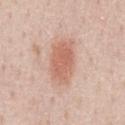Q: Was a biopsy performed?
A: catalogued during a skin exam; not biopsied
Q: Illumination type?
A: white-light
Q: How was this image acquired?
A: 15 mm crop, total-body photography
Q: Lesion location?
A: the abdomen
Q: Patient demographics?
A: male, aged 58 to 62
Q: What is the lesion's diameter?
A: about 5.5 mm